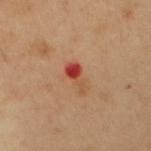<case>
<patient>
  <sex>male</sex>
  <age_approx>50</age_approx>
</patient>
<automated_metrics>
  <border_irregularity_0_10>6.0</border_irregularity_0_10>
  <color_variation_0_10>2.5</color_variation_0_10>
  <peripheral_color_asymmetry>0.5</peripheral_color_asymmetry>
</automated_metrics>
<site>abdomen</site>
<image>
  <source>total-body photography crop</source>
  <field_of_view_mm>15</field_of_view_mm>
</image>
</case>